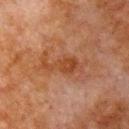Assessment: The lesion was photographed on a routine skin check and not biopsied; there is no pathology result. Image and clinical context: A region of skin cropped from a whole-body photographic capture, roughly 15 mm wide. This is a cross-polarized tile. A male subject, aged 78–82. Longest diameter approximately 5.5 mm. On the chest.